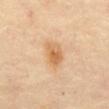Assessment:
Captured during whole-body skin photography for melanoma surveillance; the lesion was not biopsied.
Acquisition and patient details:
Located on the abdomen. The patient is a female aged around 70. A region of skin cropped from a whole-body photographic capture, roughly 15 mm wide.